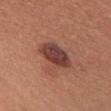Case summary:
* notes: total-body-photography surveillance lesion; no biopsy
* subject: female, aged approximately 35
* location: the upper back
* lighting: white-light
* acquisition: ~15 mm crop, total-body skin-cancer survey
* lesion diameter: about 5 mm